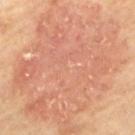An algorithmic analysis of the crop reported a lesion area of about 125 mm², an outline eccentricity of about 0.65 (0 = round, 1 = elongated), and two-axis asymmetry of about 0.25. It also reported a border-irregularity rating of about 6/10. And it measured a classifier nevus-likeness of about 75/100.
A lesion tile, about 15 mm wide, cut from a 3D total-body photograph.
Approximately 14.5 mm at its widest.
A male subject approximately 70 years of age.
Captured under cross-polarized illumination.
From the mid back.